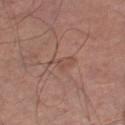Background:
An algorithmic analysis of the crop reported a lesion area of about 3.5 mm², an eccentricity of roughly 0.9, and a shape-asymmetry score of about 0.5 (0 = symmetric). The software also gave a mean CIELAB color near L≈49 a*≈21 b*≈26, roughly 6 lightness units darker than nearby skin, and a normalized lesion–skin contrast near 5. And it measured a border-irregularity index near 5.5/10, a color-variation rating of about 1/10, and radial color variation of about 0. About 3 mm across. A male patient, aged 63 to 67. A region of skin cropped from a whole-body photographic capture, roughly 15 mm wide. On the right thigh.